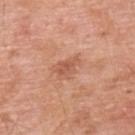Part of a total-body skin-imaging series; this lesion was reviewed on a skin check and was not flagged for biopsy.
About 2.5 mm across.
Located on the upper back.
Cropped from a whole-body photographic skin survey; the tile spans about 15 mm.
This is a white-light tile.
A male patient approximately 60 years of age.